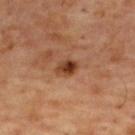Assessment:
The lesion was tiled from a total-body skin photograph and was not biopsied.
Context:
The patient is a male approximately 55 years of age. A 15 mm crop from a total-body photograph taken for skin-cancer surveillance. The lesion is located on the upper back.